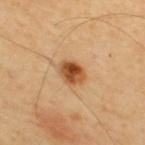Findings:
* notes: imaged on a skin check; not biopsied
* lighting: cross-polarized illumination
* site: the upper back
* diameter: ≈3 mm
* subject: male, about 65 years old
* image source: 15 mm crop, total-body photography